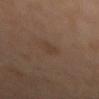notes: catalogued during a skin exam; not biopsied
automated metrics: a footprint of about 2.5 mm² and a shape-asymmetry score of about 0.3 (0 = symmetric); a nevus-likeness score of about 15/100
image source: ~15 mm tile from a whole-body skin photo
illumination: cross-polarized
patient: female, aged 48–52
size: ~2.5 mm (longest diameter)
site: the mid back